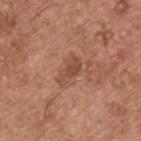Captured during whole-body skin photography for melanoma surveillance; the lesion was not biopsied. A roughly 15 mm field-of-view crop from a total-body skin photograph. On the back. Automated image analysis of the tile measured an area of roughly 5 mm² and an outline eccentricity of about 0.9 (0 = round, 1 = elongated). The software also gave an average lesion color of about L≈48 a*≈23 b*≈32 (CIELAB), about 9 CIELAB-L* units darker than the surrounding skin, and a normalized lesion–skin contrast near 6.5. The analysis additionally found a color-variation rating of about 2/10 and peripheral color asymmetry of about 0.5. And it measured an automated nevus-likeness rating near 0 out of 100 and lesion-presence confidence of about 100/100. Measured at roughly 3.5 mm in maximum diameter. This is a white-light tile. A male subject, in their mid-50s.